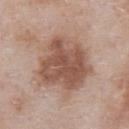Acquisition and patient details: From the abdomen. A male subject, aged 53–57. Imaged with white-light lighting. Measured at roughly 6.5 mm in maximum diameter. A close-up tile cropped from a whole-body skin photograph, about 15 mm across.